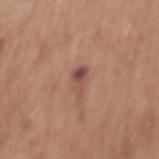Imaged during a routine full-body skin examination; the lesion was not biopsied and no histopathology is available. A male patient, aged approximately 60. About 3 mm across. This is a white-light tile. Located on the mid back. A lesion tile, about 15 mm wide, cut from a 3D total-body photograph.